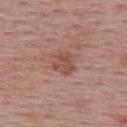biopsy_status: not biopsied; imaged during a skin examination
image:
  source: total-body photography crop
  field_of_view_mm: 15
site: upper back
lesion_size:
  long_diameter_mm_approx: 3.0
patient:
  sex: female
  age_approx: 55
lighting: white-light